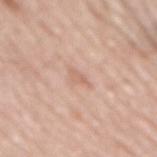Image and clinical context: Longest diameter approximately 3 mm. A 15 mm crop from a total-body photograph taken for skin-cancer surveillance. A male patient, aged around 70. From the mid back. Automated image analysis of the tile measured an average lesion color of about L≈64 a*≈20 b*≈29 (CIELAB) and a normalized border contrast of about 5. The software also gave a classifier nevus-likeness of about 0/100.